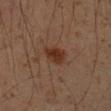workup: catalogued during a skin exam; not biopsied | subject: male, aged approximately 50 | location: the left forearm | acquisition: 15 mm crop, total-body photography.